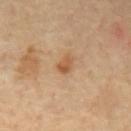Imaged during a routine full-body skin examination; the lesion was not biopsied and no histopathology is available. This image is a 15 mm lesion crop taken from a total-body photograph. The tile uses cross-polarized illumination. From the mid back. Longest diameter approximately 2.5 mm. A male patient aged 68–72. Automated image analysis of the tile measured an automated nevus-likeness rating near 25 out of 100 and a lesion-detection confidence of about 100/100.